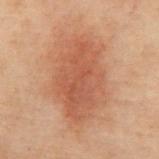{
  "biopsy_status": "not biopsied; imaged during a skin examination",
  "lighting": "cross-polarized",
  "site": "abdomen",
  "automated_metrics": {
    "cielab_L": 41,
    "cielab_a": 20,
    "cielab_b": 27,
    "vs_skin_darker_L": 8.0,
    "nevus_likeness_0_100": 100,
    "lesion_detection_confidence_0_100": 100
  },
  "patient": {
    "sex": "male",
    "age_approx": 70
  },
  "lesion_size": {
    "long_diameter_mm_approx": 8.5
  },
  "image": {
    "source": "total-body photography crop",
    "field_of_view_mm": 15
  }
}